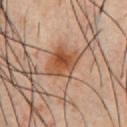The lesion-visualizer software estimated an average lesion color of about L≈49 a*≈23 b*≈34 (CIELAB), about 11 CIELAB-L* units darker than the surrounding skin, and a normalized border contrast of about 9. The subject is a male aged 38 to 42. From the chest. This is a cross-polarized tile. A region of skin cropped from a whole-body photographic capture, roughly 15 mm wide. The recorded lesion diameter is about 3.5 mm.